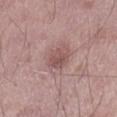Assessment: Recorded during total-body skin imaging; not selected for excision or biopsy.